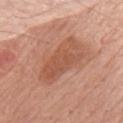{"biopsy_status": "not biopsied; imaged during a skin examination", "automated_metrics": {"cielab_L": 54, "cielab_a": 24, "cielab_b": 32, "vs_skin_darker_L": 9.0, "vs_skin_contrast_norm": 6.5, "color_variation_0_10": 3.0, "peripheral_color_asymmetry": 1.0, "nevus_likeness_0_100": 5, "lesion_detection_confidence_0_100": 100}, "site": "mid back", "patient": {"sex": "male", "age_approx": 80}, "image": {"source": "total-body photography crop", "field_of_view_mm": 15}, "lighting": "white-light", "lesion_size": {"long_diameter_mm_approx": 6.5}}